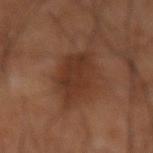Imaged during a routine full-body skin examination; the lesion was not biopsied and no histopathology is available. The lesion-visualizer software estimated a footprint of about 16 mm² and a shape-asymmetry score of about 0.2 (0 = symmetric). The analysis additionally found a mean CIELAB color near L≈29 a*≈19 b*≈26 and a lesion-to-skin contrast of about 7.5 (normalized; higher = more distinct). The software also gave border irregularity of about 2.5 on a 0–10 scale and a peripheral color-asymmetry measure near 1. It also reported an automated nevus-likeness rating near 25 out of 100 and a lesion-detection confidence of about 100/100. Measured at roughly 6 mm in maximum diameter. A close-up tile cropped from a whole-body skin photograph, about 15 mm across. The lesion is located on the mid back. A male subject, aged 58–62.